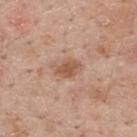* workup: no biopsy performed (imaged during a skin exam)
* lesion size: ≈3 mm
* tile lighting: white-light illumination
* location: the back
* patient: male, aged 58–62
* acquisition: total-body-photography crop, ~15 mm field of view